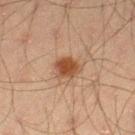Clinical impression: This lesion was catalogued during total-body skin photography and was not selected for biopsy. Image and clinical context: The tile uses cross-polarized illumination. Automated image analysis of the tile measured an area of roughly 6 mm², a shape eccentricity near 0.55, and a symmetry-axis asymmetry near 0.2. The analysis additionally found an average lesion color of about L≈40 a*≈19 b*≈29 (CIELAB) and a normalized border contrast of about 9.5. The analysis additionally found a color-variation rating of about 4/10 and peripheral color asymmetry of about 1.5. The analysis additionally found a nevus-likeness score of about 95/100 and a lesion-detection confidence of about 100/100. On the left thigh. About 3 mm across. A male patient, aged 43–47. A close-up tile cropped from a whole-body skin photograph, about 15 mm across.